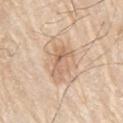| feature | finding |
|---|---|
| workup | catalogued during a skin exam; not biopsied |
| image source | total-body-photography crop, ~15 mm field of view |
| anatomic site | the right upper arm |
| patient | male, approximately 80 years of age |